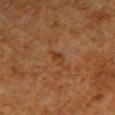On the right upper arm. A 15 mm crop from a total-body photograph taken for skin-cancer surveillance. Approximately 3 mm at its widest. The subject is a male in their 80s. Captured under cross-polarized illumination.